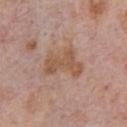| feature | finding |
|---|---|
| notes | imaged on a skin check; not biopsied |
| location | the chest |
| subject | male, approximately 75 years of age |
| acquisition | 15 mm crop, total-body photography |